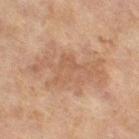<case>
<biopsy_status>not biopsied; imaged during a skin examination</biopsy_status>
<patient>
  <sex>female</sex>
  <age_approx>60</age_approx>
</patient>
<automated_metrics>
  <area_mm2_approx>33.0</area_mm2_approx>
  <eccentricity>0.8</eccentricity>
  <shape_asymmetry>0.35</shape_asymmetry>
  <nevus_likeness_0_100>0</nevus_likeness_0_100>
  <lesion_detection_confidence_0_100>100</lesion_detection_confidence_0_100>
</automated_metrics>
<image>
  <source>total-body photography crop</source>
  <field_of_view_mm>15</field_of_view_mm>
</image>
<site>leg</site>
</case>